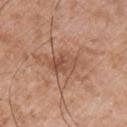The lesion was tiled from a total-body skin photograph and was not biopsied.
A male patient approximately 50 years of age.
Approximately 3.5 mm at its widest.
A close-up tile cropped from a whole-body skin photograph, about 15 mm across.
This is a white-light tile.
On the chest.